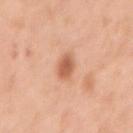<lesion>
  <biopsy_status>not biopsied; imaged during a skin examination</biopsy_status>
  <site>arm</site>
  <automated_metrics>
    <area_mm2_approx>5.0</area_mm2_approx>
    <shape_asymmetry>0.2</shape_asymmetry>
    <cielab_L>61</cielab_L>
    <cielab_a>26</cielab_a>
    <cielab_b>35</cielab_b>
    <vs_skin_darker_L>12.0</vs_skin_darker_L>
    <nevus_likeness_0_100>85</nevus_likeness_0_100>
    <lesion_detection_confidence_0_100>100</lesion_detection_confidence_0_100>
  </automated_metrics>
  <image>
    <source>total-body photography crop</source>
    <field_of_view_mm>15</field_of_view_mm>
  </image>
  <patient>
    <sex>female</sex>
    <age_approx>45</age_approx>
  </patient>
  <lighting>white-light</lighting>
</lesion>